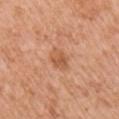Q: Is there a histopathology result?
A: catalogued during a skin exam; not biopsied
Q: Who is the patient?
A: female, aged 38–42
Q: Lesion location?
A: the right upper arm
Q: What kind of image is this?
A: 15 mm crop, total-body photography